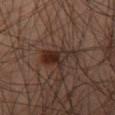tile lighting: cross-polarized
subject: male, aged around 45
acquisition: 15 mm crop, total-body photography
automated metrics: a shape-asymmetry score of about 0.4 (0 = symmetric); a lesion color around L≈26 a*≈16 b*≈21 in CIELAB, roughly 8 lightness units darker than nearby skin, and a normalized border contrast of about 9
anatomic site: the right thigh
lesion size: about 4.5 mm